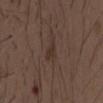{"lighting": "white-light", "patient": {"sex": "male", "age_approx": 50}, "image": {"source": "total-body photography crop", "field_of_view_mm": 15}, "site": "chest"}